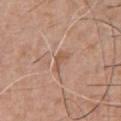Captured during whole-body skin photography for melanoma surveillance; the lesion was not biopsied.
A roughly 15 mm field-of-view crop from a total-body skin photograph.
The patient is a male aged around 60.
Imaged with white-light lighting.
Approximately 2.5 mm at its widest.
The lesion is on the chest.